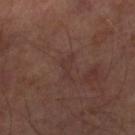| field | value |
|---|---|
| workup | imaged on a skin check; not biopsied |
| patient | male, aged 63 to 67 |
| image source | 15 mm crop, total-body photography |
| diameter | ~3 mm (longest diameter) |
| tile lighting | cross-polarized |
| body site | the right lower leg |
| automated lesion analysis | an average lesion color of about L≈31 a*≈18 b*≈19 (CIELAB), a lesion–skin lightness drop of about 4, and a normalized lesion–skin contrast near 4.5; a border-irregularity index near 6.5/10 |